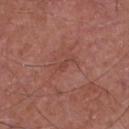Part of a total-body skin-imaging series; this lesion was reviewed on a skin check and was not flagged for biopsy.
Automated image analysis of the tile measured a border-irregularity rating of about 6/10, a color-variation rating of about 0/10, and a peripheral color-asymmetry measure near 0.
Captured under white-light illumination.
On the chest.
The patient is a male aged approximately 65.
This image is a 15 mm lesion crop taken from a total-body photograph.
About 2.5 mm across.